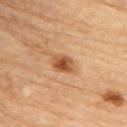Impression: The lesion was photographed on a routine skin check and not biopsied; there is no pathology result. Context: A male subject, aged 83 or older. Located on the upper back. A region of skin cropped from a whole-body photographic capture, roughly 15 mm wide.